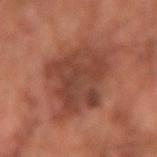<case>
  <biopsy_status>not biopsied; imaged during a skin examination</biopsy_status>
  <lesion_size>
    <long_diameter_mm_approx>9.5</long_diameter_mm_approx>
  </lesion_size>
  <patient>
    <sex>male</sex>
    <age_approx>65</age_approx>
  </patient>
  <image>
    <source>total-body photography crop</source>
    <field_of_view_mm>15</field_of_view_mm>
  </image>
  <lighting>cross-polarized</lighting>
  <site>left forearm</site>
</case>